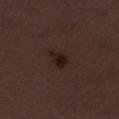Imaged during a routine full-body skin examination; the lesion was not biopsied and no histopathology is available.
Approximately 3 mm at its widest.
This image is a 15 mm lesion crop taken from a total-body photograph.
This is a white-light tile.
The subject is a female aged 48–52.
Located on the left lower leg.